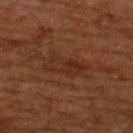The lesion was photographed on a routine skin check and not biopsied; there is no pathology result. The lesion is located on the upper back. Imaged with cross-polarized lighting. Cropped from a total-body skin-imaging series; the visible field is about 15 mm. A male patient aged approximately 65. The lesion's longest dimension is about 5 mm.